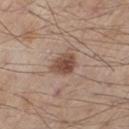{"biopsy_status": "not biopsied; imaged during a skin examination", "automated_metrics": {"cielab_L": 48, "cielab_a": 18, "cielab_b": 26, "vs_skin_darker_L": 13.0, "vs_skin_contrast_norm": 9.0, "border_irregularity_0_10": 2.0, "color_variation_0_10": 4.0, "peripheral_color_asymmetry": 1.5, "nevus_likeness_0_100": 95, "lesion_detection_confidence_0_100": 100}, "site": "chest", "patient": {"sex": "male", "age_approx": 80}, "lesion_size": {"long_diameter_mm_approx": 3.0}, "lighting": "white-light", "image": {"source": "total-body photography crop", "field_of_view_mm": 15}}